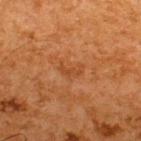follow-up: imaged on a skin check; not biopsied
automated metrics: a border-irregularity rating of about 6/10, internal color variation of about 0 on a 0–10 scale, and peripheral color asymmetry of about 0; an automated nevus-likeness rating near 0 out of 100 and a lesion-detection confidence of about 100/100
tile lighting: cross-polarized
image: total-body-photography crop, ~15 mm field of view
body site: the upper back
size: ≈3 mm
subject: male, roughly 65 years of age